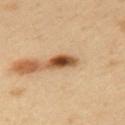The recorded lesion diameter is about 3.5 mm.
Located on the left upper arm.
Automated tile analysis of the lesion measured a lesion area of about 5 mm², an eccentricity of roughly 0.85, and a shape-asymmetry score of about 0.25 (0 = symmetric). And it measured a mean CIELAB color near L≈51 a*≈22 b*≈38 and a normalized border contrast of about 13. The analysis additionally found a border-irregularity rating of about 3/10, a color-variation rating of about 7/10, and a peripheral color-asymmetry measure near 2. The software also gave a nevus-likeness score of about 100/100 and a detector confidence of about 100 out of 100 that the crop contains a lesion.
This image is a 15 mm lesion crop taken from a total-body photograph.
A male subject about 40 years old.
This is a cross-polarized tile.